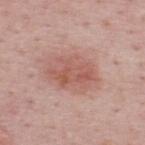Findings:
* notes: catalogued during a skin exam; not biopsied
* lesion diameter: ≈5.5 mm
* imaging modality: ~15 mm crop, total-body skin-cancer survey
* tile lighting: white-light
* subject: male, roughly 50 years of age
* anatomic site: the upper back
* automated metrics: a mean CIELAB color near L≈56 a*≈24 b*≈26, about 9 CIELAB-L* units darker than the surrounding skin, and a normalized border contrast of about 6.5; lesion-presence confidence of about 100/100